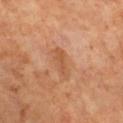workup: imaged on a skin check; not biopsied | TBP lesion metrics: an area of roughly 4 mm² and a shape eccentricity near 0.95; an average lesion color of about L≈53 a*≈24 b*≈36 (CIELAB) and a lesion-to-skin contrast of about 6 (normalized; higher = more distinct); border irregularity of about 4 on a 0–10 scale, internal color variation of about 1 on a 0–10 scale, and radial color variation of about 0.5 | anatomic site: the chest | lesion diameter: about 4 mm | lighting: cross-polarized illumination | subject: female, about 65 years old | image source: ~15 mm crop, total-body skin-cancer survey.